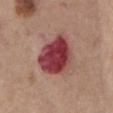biopsy_status: not biopsied; imaged during a skin examination
patient:
  sex: male
  age_approx: 75
image:
  source: total-body photography crop
  field_of_view_mm: 15
lighting: white-light
automated_metrics:
  cielab_L: 41
  cielab_a: 32
  cielab_b: 21
  vs_skin_darker_L: 17.0
  vs_skin_contrast_norm: 13.0
  nevus_likeness_0_100: 0
  lesion_detection_confidence_0_100: 100
site: chest
lesion_size:
  long_diameter_mm_approx: 5.5